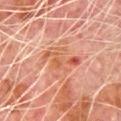follow-up — no biopsy performed (imaged during a skin exam) | lesion size — ≈4.5 mm | patient — male, aged 78 to 82 | image — total-body-photography crop, ~15 mm field of view | location — the chest.